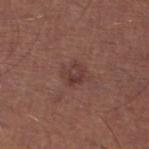biopsy status: total-body-photography surveillance lesion; no biopsy
subject: male, aged 63–67
diameter: about 2.5 mm
site: the left lower leg
tile lighting: white-light illumination
acquisition: 15 mm crop, total-body photography